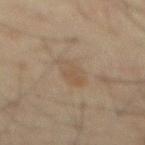workup=catalogued during a skin exam; not biopsied
lighting=cross-polarized
image=15 mm crop, total-body photography
lesion size=≈4 mm
TBP lesion metrics=border irregularity of about 3 on a 0–10 scale, a within-lesion color-variation index near 3/10, and peripheral color asymmetry of about 1
subject=male, aged 58 to 62